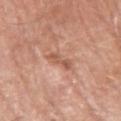The lesion was tiled from a total-body skin photograph and was not biopsied.
From the right upper arm.
A close-up tile cropped from a whole-body skin photograph, about 15 mm across.
A male subject, aged around 80.